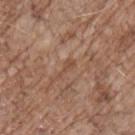Imaged during a routine full-body skin examination; the lesion was not biopsied and no histopathology is available.
A 15 mm crop from a total-body photograph taken for skin-cancer surveillance.
From the chest.
This is a white-light tile.
Measured at roughly 2.5 mm in maximum diameter.
The total-body-photography lesion software estimated border irregularity of about 6.5 on a 0–10 scale, a within-lesion color-variation index near 0/10, and a peripheral color-asymmetry measure near 0. The software also gave a classifier nevus-likeness of about 0/100.
A male subject, aged 68–72.